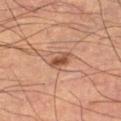biopsy_status: not biopsied; imaged during a skin examination
lighting: cross-polarized
lesion_size:
  long_diameter_mm_approx: 3.0
automated_metrics:
  cielab_L: 45
  cielab_a: 21
  cielab_b: 29
  vs_skin_contrast_norm: 8.0
  nevus_likeness_0_100: 85
  lesion_detection_confidence_0_100: 100
site: leg
image:
  source: total-body photography crop
  field_of_view_mm: 15
patient:
  sex: male
  age_approx: 55